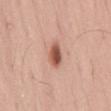follow-up = imaged on a skin check; not biopsied | site = the lower back | image source = 15 mm crop, total-body photography | subject = male, aged 33–37.